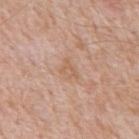biopsy status = no biopsy performed (imaged during a skin exam)
image = ~15 mm crop, total-body skin-cancer survey
lighting = white-light illumination
patient = male, aged 48 to 52
anatomic site = the back
automated lesion analysis = a lesion area of about 3 mm² and an eccentricity of roughly 0.75; internal color variation of about 1.5 on a 0–10 scale and a peripheral color-asymmetry measure near 0.5; a detector confidence of about 100 out of 100 that the crop contains a lesion